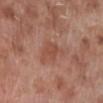Impression:
Captured during whole-body skin photography for melanoma surveillance; the lesion was not biopsied.
Context:
The lesion is on the leg. Imaged with white-light lighting. Automated tile analysis of the lesion measured a border-irregularity rating of about 2/10, a color-variation rating of about 2/10, and peripheral color asymmetry of about 0.5. The analysis additionally found a lesion-detection confidence of about 100/100. Cropped from a whole-body photographic skin survey; the tile spans about 15 mm. A male subject, aged around 70.